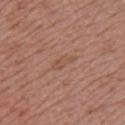{"biopsy_status": "not biopsied; imaged during a skin examination", "site": "front of the torso", "image": {"source": "total-body photography crop", "field_of_view_mm": 15}, "patient": {"sex": "female", "age_approx": 50}}